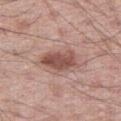Part of a total-body skin-imaging series; this lesion was reviewed on a skin check and was not flagged for biopsy. The total-body-photography lesion software estimated a border-irregularity rating of about 2.5/10, a within-lesion color-variation index near 3.5/10, and radial color variation of about 1.5. The analysis additionally found an automated nevus-likeness rating near 100 out of 100 and a lesion-detection confidence of about 100/100. A 15 mm close-up extracted from a 3D total-body photography capture. The lesion's longest dimension is about 4.5 mm. A male subject approximately 60 years of age. From the leg.